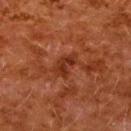{"biopsy_status": "not biopsied; imaged during a skin examination", "image": {"source": "total-body photography crop", "field_of_view_mm": 15}, "site": "back", "patient": {"sex": "female", "age_approx": 50}}